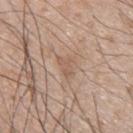Assessment: No biopsy was performed on this lesion — it was imaged during a full skin examination and was not determined to be concerning. Context: The total-body-photography lesion software estimated a lesion area of about 3.5 mm², an eccentricity of roughly 0.7, and two-axis asymmetry of about 0.4. And it measured an average lesion color of about L≈57 a*≈17 b*≈29 (CIELAB) and a normalized lesion–skin contrast near 5. A male patient roughly 45 years of age. Cropped from a total-body skin-imaging series; the visible field is about 15 mm. From the back. Captured under white-light illumination. Measured at roughly 2.5 mm in maximum diameter.